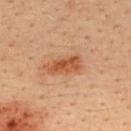Q: Is there a histopathology result?
A: no biopsy performed (imaged during a skin exam)
Q: What lighting was used for the tile?
A: cross-polarized illumination
Q: Where on the body is the lesion?
A: the upper back
Q: Patient demographics?
A: male, in their mid- to late 30s
Q: Lesion size?
A: ≈4.5 mm
Q: What kind of image is this?
A: 15 mm crop, total-body photography